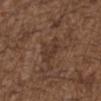follow-up: imaged on a skin check; not biopsied
illumination: white-light illumination
diameter: ~3.5 mm (longest diameter)
TBP lesion metrics: a lesion area of about 6 mm² and an eccentricity of roughly 0.65; an average lesion color of about L≈35 a*≈17 b*≈25 (CIELAB), roughly 6 lightness units darker than nearby skin, and a normalized lesion–skin contrast near 5.5; a classifier nevus-likeness of about 0/100 and a detector confidence of about 85 out of 100 that the crop contains a lesion
subject: male, about 50 years old
image source: ~15 mm crop, total-body skin-cancer survey
anatomic site: the front of the torso